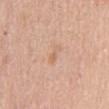Findings:
- notes — total-body-photography surveillance lesion; no biopsy
- image — total-body-photography crop, ~15 mm field of view
- illumination — white-light illumination
- anatomic site — the chest
- subject — female, approximately 65 years of age